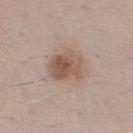workup: catalogued during a skin exam; not biopsied | body site: the leg | lesion diameter: ~4 mm (longest diameter) | patient: female, aged around 40 | image-analysis metrics: two-axis asymmetry of about 0.2; a border-irregularity index near 2/10 and a color-variation rating of about 5/10; a classifier nevus-likeness of about 80/100 and a detector confidence of about 100 out of 100 that the crop contains a lesion | lighting: white-light | image source: total-body-photography crop, ~15 mm field of view.